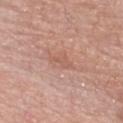image: ~15 mm tile from a whole-body skin photo | image-analysis metrics: a lesion area of about 3 mm², a shape eccentricity near 0.9, and a shape-asymmetry score of about 0.35 (0 = symmetric); a border-irregularity index near 4/10, internal color variation of about 0.5 on a 0–10 scale, and radial color variation of about 0 | subject: male, roughly 75 years of age | anatomic site: the chest | lesion diameter: about 3 mm.